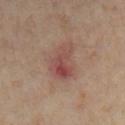workup: catalogued during a skin exam; not biopsied
anatomic site: the right lower leg
acquisition: total-body-photography crop, ~15 mm field of view
TBP lesion metrics: a border-irregularity index near 3.5/10 and a color-variation rating of about 7.5/10
patient: female, approximately 35 years of age